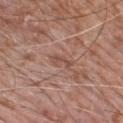Findings:
– follow-up · catalogued during a skin exam; not biopsied
– location · the left lower leg
– tile lighting · white-light illumination
– acquisition · 15 mm crop, total-body photography
– patient · male, approximately 75 years of age